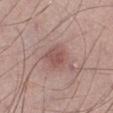Recorded during total-body skin imaging; not selected for excision or biopsy.
A male subject, aged around 50.
This image is a 15 mm lesion crop taken from a total-body photograph.
The lesion is on the right lower leg.
The tile uses white-light illumination.
An algorithmic analysis of the crop reported a mean CIELAB color near L≈52 a*≈21 b*≈22, about 9 CIELAB-L* units darker than the surrounding skin, and a lesion-to-skin contrast of about 6.5 (normalized; higher = more distinct).